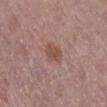Impression: Imaged during a routine full-body skin examination; the lesion was not biopsied and no histopathology is available. Image and clinical context: Cropped from a total-body skin-imaging series; the visible field is about 15 mm. Automated image analysis of the tile measured a footprint of about 4.5 mm² and a shape eccentricity near 0.7. And it measured an average lesion color of about L≈49 a*≈22 b*≈24 (CIELAB), about 8 CIELAB-L* units darker than the surrounding skin, and a normalized border contrast of about 6.5. The tile uses white-light illumination. Longest diameter approximately 3 mm. The lesion is on the right lower leg. A female subject, aged approximately 65.